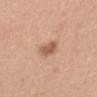The lesion was photographed on a routine skin check and not biopsied; there is no pathology result. Located on the abdomen. Cropped from a total-body skin-imaging series; the visible field is about 15 mm. A female subject, aged 53 to 57. The lesion's longest dimension is about 2.5 mm. Imaged with white-light lighting.